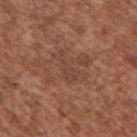Captured during whole-body skin photography for melanoma surveillance; the lesion was not biopsied.